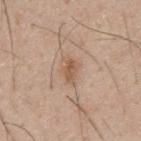Imaged during a routine full-body skin examination; the lesion was not biopsied and no histopathology is available. The recorded lesion diameter is about 2.5 mm. The lesion is located on the upper back. The subject is a male about 30 years old. A region of skin cropped from a whole-body photographic capture, roughly 15 mm wide.